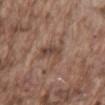<lesion>
  <biopsy_status>not biopsied; imaged during a skin examination</biopsy_status>
  <image>
    <source>total-body photography crop</source>
    <field_of_view_mm>15</field_of_view_mm>
  </image>
  <lighting>white-light</lighting>
  <automated_metrics>
    <cielab_L>45</cielab_L>
    <cielab_a>17</cielab_a>
    <cielab_b>25</cielab_b>
    <vs_skin_darker_L>8.0</vs_skin_darker_L>
    <vs_skin_contrast_norm>6.5</vs_skin_contrast_norm>
    <color_variation_0_10>4.0</color_variation_0_10>
    <nevus_likeness_0_100>5</nevus_likeness_0_100>
    <lesion_detection_confidence_0_100>95</lesion_detection_confidence_0_100>
  </automated_metrics>
  <site>lower back</site>
  <lesion_size>
    <long_diameter_mm_approx>4.0</long_diameter_mm_approx>
  </lesion_size>
  <patient>
    <sex>male</sex>
    <age_approx>75</age_approx>
  </patient>
</lesion>